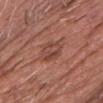Impression:
Recorded during total-body skin imaging; not selected for excision or biopsy.
Image and clinical context:
Longest diameter approximately 3.5 mm. Cropped from a total-body skin-imaging series; the visible field is about 15 mm. The subject is a male roughly 65 years of age. The lesion is located on the head or neck.